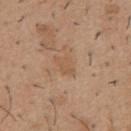biopsy status = no biopsy performed (imaged during a skin exam)
body site = the mid back
imaging modality = 15 mm crop, total-body photography
illumination = white-light
diameter = about 2.5 mm
automated metrics = a shape eccentricity near 0.75; an average lesion color of about L≈55 a*≈18 b*≈32 (CIELAB), about 6 CIELAB-L* units darker than the surrounding skin, and a normalized border contrast of about 4.5; a border-irregularity rating of about 2.5/10, a color-variation rating of about 1/10, and radial color variation of about 0.5; a nevus-likeness score of about 0/100
patient = male, aged 38–42